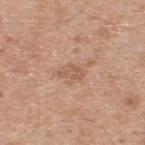Captured during whole-body skin photography for melanoma surveillance; the lesion was not biopsied.
Automated image analysis of the tile measured a footprint of about 3 mm², a shape eccentricity near 0.85, and a symmetry-axis asymmetry near 0.4. The software also gave an average lesion color of about L≈57 a*≈20 b*≈31 (CIELAB), about 8 CIELAB-L* units darker than the surrounding skin, and a normalized border contrast of about 5.5. The analysis additionally found a border-irregularity rating of about 4.5/10, a color-variation rating of about 1/10, and peripheral color asymmetry of about 0.5.
A close-up tile cropped from a whole-body skin photograph, about 15 mm across.
A male patient about 55 years old.
The tile uses white-light illumination.
From the upper back.